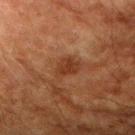Clinical impression: Captured during whole-body skin photography for melanoma surveillance; the lesion was not biopsied. Context: Approximately 3 mm at its widest. A male subject aged 63 to 67. Located on the right upper arm. The lesion-visualizer software estimated a footprint of about 5.5 mm², an outline eccentricity of about 0.7 (0 = round, 1 = elongated), and two-axis asymmetry of about 0.3. The analysis additionally found an average lesion color of about L≈30 a*≈21 b*≈28 (CIELAB) and a normalized border contrast of about 7.5. It also reported a border-irregularity index near 3.5/10 and a peripheral color-asymmetry measure near 0.5. It also reported a classifier nevus-likeness of about 70/100. Imaged with cross-polarized lighting. A lesion tile, about 15 mm wide, cut from a 3D total-body photograph.